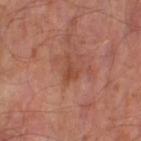• notes — no biopsy performed (imaged during a skin exam)
• diameter — ~3 mm (longest diameter)
• anatomic site — the left thigh
• image source — 15 mm crop, total-body photography
• lighting — cross-polarized
• subject — male, aged 63–67
• image-analysis metrics — a shape-asymmetry score of about 0.55 (0 = symmetric)